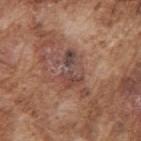The lesion's longest dimension is about 4 mm. Cropped from a total-body skin-imaging series; the visible field is about 15 mm. The tile uses white-light illumination. The subject is a male aged 73 to 77. The lesion is located on the arm.